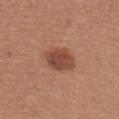lighting: white-light illumination | anatomic site: the front of the torso | patient: female, approximately 25 years of age | automated lesion analysis: a footprint of about 11 mm², an outline eccentricity of about 0.6 (0 = round, 1 = elongated), and a shape-asymmetry score of about 0.15 (0 = symmetric); a lesion–skin lightness drop of about 10 and a lesion-to-skin contrast of about 7.5 (normalized; higher = more distinct) | image: 15 mm crop, total-body photography.